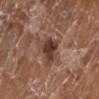<tbp_lesion>
  <biopsy_status>not biopsied; imaged during a skin examination</biopsy_status>
  <automated_metrics>
    <color_variation_0_10>3.0</color_variation_0_10>
    <peripheral_color_asymmetry>1.0</peripheral_color_asymmetry>
  </automated_metrics>
  <image>
    <source>total-body photography crop</source>
    <field_of_view_mm>15</field_of_view_mm>
  </image>
  <patient>
    <sex>female</sex>
    <age_approx>75</age_approx>
  </patient>
  <site>left lower leg</site>
</tbp_lesion>